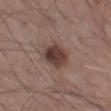Captured during whole-body skin photography for melanoma surveillance; the lesion was not biopsied.
A male patient about 60 years old.
A roughly 15 mm field-of-view crop from a total-body skin photograph.
On the left thigh.